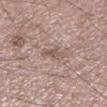Captured during whole-body skin photography for melanoma surveillance; the lesion was not biopsied.
A male patient, aged 48 to 52.
A roughly 15 mm field-of-view crop from a total-body skin photograph.
The recorded lesion diameter is about 3 mm.
Captured under white-light illumination.
Automated tile analysis of the lesion measured a lesion-to-skin contrast of about 6 (normalized; higher = more distinct). And it measured border irregularity of about 3.5 on a 0–10 scale, a within-lesion color-variation index near 1.5/10, and peripheral color asymmetry of about 0.5.
The lesion is located on the left lower leg.